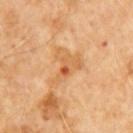<tbp_lesion>
<biopsy_status>not biopsied; imaged during a skin examination</biopsy_status>
<patient>
  <sex>male</sex>
  <age_approx>65</age_approx>
</patient>
<lighting>cross-polarized</lighting>
<lesion_size>
  <long_diameter_mm_approx>5.0</long_diameter_mm_approx>
</lesion_size>
<image>
  <source>total-body photography crop</source>
  <field_of_view_mm>15</field_of_view_mm>
</image>
<automated_metrics>
  <cielab_L>61</cielab_L>
  <cielab_a>23</cielab_a>
  <cielab_b>42</cielab_b>
  <vs_skin_darker_L>8.0</vs_skin_darker_L>
  <vs_skin_contrast_norm>6.0</vs_skin_contrast_norm>
  <border_irregularity_0_10>7.0</border_irregularity_0_10>
  <color_variation_0_10>9.5</color_variation_0_10>
  <peripheral_color_asymmetry>4.0</peripheral_color_asymmetry>
  <lesion_detection_confidence_0_100>100</lesion_detection_confidence_0_100>
</automated_metrics>
</tbp_lesion>